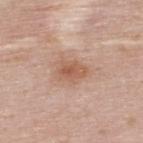{
  "biopsy_status": "not biopsied; imaged during a skin examination",
  "image": {
    "source": "total-body photography crop",
    "field_of_view_mm": 15
  },
  "site": "upper back",
  "patient": {
    "sex": "female",
    "age_approx": 50
  },
  "lesion_size": {
    "long_diameter_mm_approx": 3.5
  },
  "automated_metrics": {
    "cielab_L": 58,
    "cielab_a": 21,
    "cielab_b": 30,
    "vs_skin_darker_L": 9.0,
    "vs_skin_contrast_norm": 7.0,
    "border_irregularity_0_10": 2.0,
    "color_variation_0_10": 3.0,
    "peripheral_color_asymmetry": 1.0
  },
  "lighting": "white-light"
}